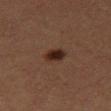• biopsy status: no biopsy performed (imaged during a skin exam)
• location: the right thigh
• image: total-body-photography crop, ~15 mm field of view
• automated metrics: a mean CIELAB color near L≈21 a*≈15 b*≈20, a lesion–skin lightness drop of about 9, and a lesion-to-skin contrast of about 11 (normalized; higher = more distinct); border irregularity of about 2 on a 0–10 scale and a color-variation rating of about 4/10
• lesion diameter: about 2.5 mm
• patient: female, approximately 40 years of age